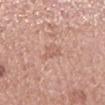{"site": "back", "image": {"source": "total-body photography crop", "field_of_view_mm": 15}, "lesion_size": {"long_diameter_mm_approx": 3.0}, "patient": {"sex": "male", "age_approx": 65}}